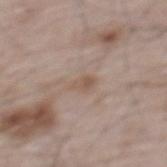notes = imaged on a skin check; not biopsied
lighting = white-light
site = the mid back
patient = male, roughly 65 years of age
acquisition = ~15 mm crop, total-body skin-cancer survey
size = about 2.5 mm